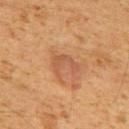  biopsy_status: not biopsied; imaged during a skin examination
  patient:
    sex: male
    age_approx: 60
  site: upper back
  image:
    source: total-body photography crop
    field_of_view_mm: 15